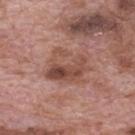Findings:
– notes — catalogued during a skin exam; not biopsied
– anatomic site — the mid back
– TBP lesion metrics — a footprint of about 15 mm², an outline eccentricity of about 0.65 (0 = round, 1 = elongated), and a shape-asymmetry score of about 0.3 (0 = symmetric); a lesion–skin lightness drop of about 9 and a lesion-to-skin contrast of about 7 (normalized; higher = more distinct); an automated nevus-likeness rating near 0 out of 100 and lesion-presence confidence of about 100/100
– lighting — white-light
– subject — male, roughly 70 years of age
– image — ~15 mm tile from a whole-body skin photo
– lesion size — ≈5 mm